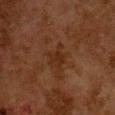Recorded during total-body skin imaging; not selected for excision or biopsy.
A lesion tile, about 15 mm wide, cut from a 3D total-body photograph.
A female subject, approximately 50 years of age.
From the chest.
The total-body-photography lesion software estimated a footprint of about 4.5 mm², an outline eccentricity of about 0.65 (0 = round, 1 = elongated), and two-axis asymmetry of about 0.4. The analysis additionally found a mean CIELAB color near L≈22 a*≈17 b*≈26, a lesion–skin lightness drop of about 5, and a lesion-to-skin contrast of about 6 (normalized; higher = more distinct). It also reported a nevus-likeness score of about 0/100.
Approximately 3 mm at its widest.